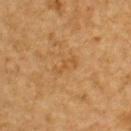Captured during whole-body skin photography for melanoma surveillance; the lesion was not biopsied. From the back. Automated tile analysis of the lesion measured an area of roughly 2.5 mm² and a shape eccentricity near 0.9. And it measured a lesion color around L≈46 a*≈19 b*≈37 in CIELAB and a lesion-to-skin contrast of about 4.5 (normalized; higher = more distinct). It also reported a nevus-likeness score of about 0/100 and lesion-presence confidence of about 100/100. This is a cross-polarized tile. The patient is a male roughly 85 years of age or older. A lesion tile, about 15 mm wide, cut from a 3D total-body photograph.